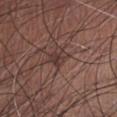{"biopsy_status": "not biopsied; imaged during a skin examination", "automated_metrics": {"area_mm2_approx": 4.0, "eccentricity": 0.7, "shape_asymmetry": 0.4, "cielab_L": 36, "cielab_a": 18, "cielab_b": 20, "vs_skin_contrast_norm": 7.0, "border_irregularity_0_10": 3.5, "color_variation_0_10": 2.0, "peripheral_color_asymmetry": 1.0, "nevus_likeness_0_100": 0, "lesion_detection_confidence_0_100": 65}, "site": "abdomen", "image": {"source": "total-body photography crop", "field_of_view_mm": 15}, "patient": {"sex": "male", "age_approx": 75}, "lesion_size": {"long_diameter_mm_approx": 2.5}, "lighting": "white-light"}